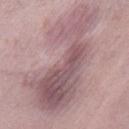| field | value |
|---|---|
| workup | total-body-photography surveillance lesion; no biopsy |
| TBP lesion metrics | a footprint of about 60 mm² and a shape eccentricity near 0.9; a border-irregularity rating of about 6.5/10, internal color variation of about 7 on a 0–10 scale, and a peripheral color-asymmetry measure near 2.5; a lesion-detection confidence of about 5/100 |
| body site | the right thigh |
| subject | female, aged 38 to 42 |
| lesion diameter | ~13.5 mm (longest diameter) |
| imaging modality | total-body-photography crop, ~15 mm field of view |